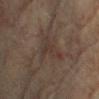site: upper back
image:
  source: total-body photography crop
  field_of_view_mm: 15
patient:
  sex: female
  age_approx: 80